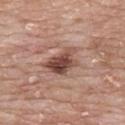Clinical impression:
Captured during whole-body skin photography for melanoma surveillance; the lesion was not biopsied.
Background:
Automated tile analysis of the lesion measured an area of roughly 9 mm², an eccentricity of roughly 0.8, and two-axis asymmetry of about 0.35. And it measured internal color variation of about 6 on a 0–10 scale. The lesion is on the chest. A 15 mm close-up extracted from a 3D total-body photography capture. Captured under white-light illumination. A female patient, aged approximately 65. Longest diameter approximately 4.5 mm.